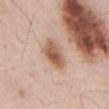Captured during whole-body skin photography for melanoma surveillance; the lesion was not biopsied.
The recorded lesion diameter is about 4 mm.
A male subject, about 55 years old.
The lesion is on the abdomen.
The lesion-visualizer software estimated a footprint of about 9 mm² and an outline eccentricity of about 0.8 (0 = round, 1 = elongated). The software also gave border irregularity of about 2 on a 0–10 scale, internal color variation of about 6 on a 0–10 scale, and radial color variation of about 1.5.
A lesion tile, about 15 mm wide, cut from a 3D total-body photograph.